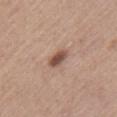follow-up: imaged on a skin check; not biopsied | body site: the left upper arm | lighting: white-light | image source: ~15 mm crop, total-body skin-cancer survey | automated metrics: a footprint of about 4.5 mm², a shape eccentricity near 0.7, and a shape-asymmetry score of about 0.25 (0 = symmetric) | patient: female, about 40 years old.